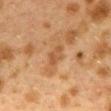workup: catalogued during a skin exam; not biopsied | lesion diameter: about 2.5 mm | anatomic site: the back | image source: 15 mm crop, total-body photography | patient: female, about 40 years old.